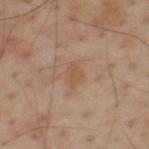Impression: This lesion was catalogued during total-body skin photography and was not selected for biopsy. Acquisition and patient details: A male subject aged 53 to 57. The tile uses cross-polarized illumination. Cropped from a total-body skin-imaging series; the visible field is about 15 mm. Located on the upper back. Automated image analysis of the tile measured a classifier nevus-likeness of about 0/100 and lesion-presence confidence of about 100/100.